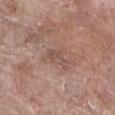Captured during whole-body skin photography for melanoma surveillance; the lesion was not biopsied. About 3.5 mm across. The tile uses white-light illumination. Cropped from a whole-body photographic skin survey; the tile spans about 15 mm. The subject is a male aged approximately 80. Located on the abdomen.